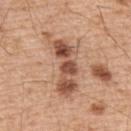Findings:
– follow-up · no biopsy performed (imaged during a skin exam)
– patient · male, in their mid- to late 50s
– body site · the upper back
– image-analysis metrics · a lesion color around L≈53 a*≈22 b*≈31 in CIELAB, about 14 CIELAB-L* units darker than the surrounding skin, and a lesion-to-skin contrast of about 9 (normalized; higher = more distinct)
– image · 15 mm crop, total-body photography
– tile lighting · white-light illumination